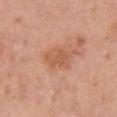On the chest. This is a white-light tile. Automated tile analysis of the lesion measured a lesion area of about 7 mm², a shape eccentricity near 0.75, and a shape-asymmetry score of about 0.25 (0 = symmetric). The software also gave about 8 CIELAB-L* units darker than the surrounding skin and a lesion-to-skin contrast of about 6 (normalized; higher = more distinct). And it measured border irregularity of about 2.5 on a 0–10 scale, a color-variation rating of about 2.5/10, and a peripheral color-asymmetry measure near 1. Cropped from a total-body skin-imaging series; the visible field is about 15 mm. The patient is a female roughly 60 years of age. Approximately 3.5 mm at its widest.